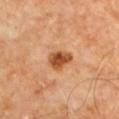workup: imaged on a skin check; not biopsied | patient: male, aged 58–62 | anatomic site: the upper back | acquisition: 15 mm crop, total-body photography | TBP lesion metrics: an average lesion color of about L≈49 a*≈25 b*≈39 (CIELAB), a lesion–skin lightness drop of about 14, and a normalized border contrast of about 10; an automated nevus-likeness rating near 95 out of 100.